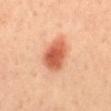| key | value |
|---|---|
| workup | total-body-photography surveillance lesion; no biopsy |
| TBP lesion metrics | an area of roughly 12 mm², an eccentricity of roughly 0.7, and a shape-asymmetry score of about 0.2 (0 = symmetric); a nevus-likeness score of about 100/100 and a lesion-detection confidence of about 100/100 |
| tile lighting | cross-polarized illumination |
| diameter | ≈4.5 mm |
| subject | male, aged around 40 |
| body site | the mid back |
| image | ~15 mm crop, total-body skin-cancer survey |